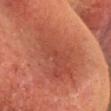illumination = cross-polarized illumination
diameter = ≈8 mm
image = ~15 mm crop, total-body skin-cancer survey
anatomic site = the head or neck
patient = female, approximately 55 years of age